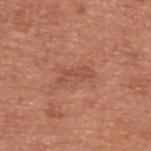{"biopsy_status": "not biopsied; imaged during a skin examination"}